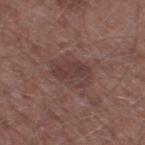This lesion was catalogued during total-body skin photography and was not selected for biopsy.
A lesion tile, about 15 mm wide, cut from a 3D total-body photograph.
An algorithmic analysis of the crop reported an area of roughly 14 mm² and an eccentricity of roughly 0.75. It also reported a color-variation rating of about 3.5/10 and a peripheral color-asymmetry measure near 1. And it measured an automated nevus-likeness rating near 0 out of 100.
A male subject about 60 years old.
The recorded lesion diameter is about 5.5 mm.
The lesion is located on the leg.